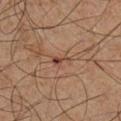Impression: Captured during whole-body skin photography for melanoma surveillance; the lesion was not biopsied.